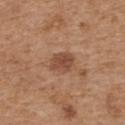Impression: The lesion was tiled from a total-body skin photograph and was not biopsied. Background: About 3 mm across. The patient is a male approximately 70 years of age. A region of skin cropped from a whole-body photographic capture, roughly 15 mm wide. From the upper back. Automated tile analysis of the lesion measured a border-irregularity index near 2.5/10, a color-variation rating of about 2.5/10, and radial color variation of about 1.